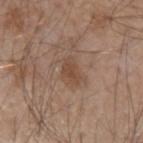Part of a total-body skin-imaging series; this lesion was reviewed on a skin check and was not flagged for biopsy.
About 3 mm across.
The lesion is located on the left forearm.
This is a white-light tile.
A 15 mm close-up extracted from a 3D total-body photography capture.
Automated image analysis of the tile measured a mean CIELAB color near L≈46 a*≈18 b*≈28, roughly 8 lightness units darker than nearby skin, and a normalized lesion–skin contrast near 6.5. The analysis additionally found a border-irregularity rating of about 3.5/10 and a color-variation rating of about 1/10. The analysis additionally found a nevus-likeness score of about 5/100 and a lesion-detection confidence of about 100/100.
A male patient roughly 55 years of age.